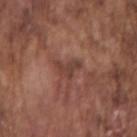– follow-up: catalogued during a skin exam; not biopsied
– patient: male, roughly 75 years of age
– size: ≈3 mm
– acquisition: ~15 mm crop, total-body skin-cancer survey
– location: the right upper arm